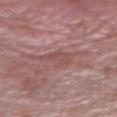Imaged during a routine full-body skin examination; the lesion was not biopsied and no histopathology is available.
A region of skin cropped from a whole-body photographic capture, roughly 15 mm wide.
A male patient, aged 38 to 42.
Automated tile analysis of the lesion measured an eccentricity of roughly 0.8 and a shape-asymmetry score of about 0.35 (0 = symmetric).
This is a white-light tile.
About 4 mm across.
From the right forearm.